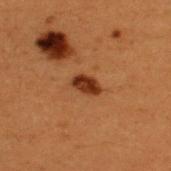{
  "biopsy_status": "not biopsied; imaged during a skin examination",
  "patient": {
    "sex": "male",
    "age_approx": 50
  },
  "lesion_size": {
    "long_diameter_mm_approx": 3.0
  },
  "automated_metrics": {
    "area_mm2_approx": 5.0,
    "shape_asymmetry": 0.2,
    "cielab_L": 29,
    "cielab_a": 21,
    "cielab_b": 30,
    "vs_skin_darker_L": 11.0,
    "vs_skin_contrast_norm": 11.0,
    "lesion_detection_confidence_0_100": 100
  },
  "site": "upper back",
  "image": {
    "source": "total-body photography crop",
    "field_of_view_mm": 15
  }
}